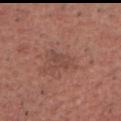Clinical impression:
Imaged during a routine full-body skin examination; the lesion was not biopsied and no histopathology is available.
Image and clinical context:
The patient is a male roughly 65 years of age. Longest diameter approximately 2.5 mm. A 15 mm close-up extracted from a 3D total-body photography capture. The lesion is located on the head or neck. Captured under white-light illumination.